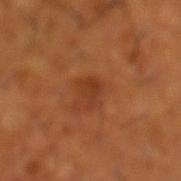biopsy status: catalogued during a skin exam; not biopsied
size: about 2.5 mm
automated metrics: an average lesion color of about L≈38 a*≈27 b*≈37 (CIELAB), about 7 CIELAB-L* units darker than the surrounding skin, and a normalized lesion–skin contrast near 6
imaging modality: 15 mm crop, total-body photography
patient: male, aged around 65
site: the leg